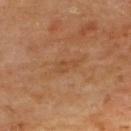follow-up: imaged on a skin check; not biopsied
illumination: cross-polarized illumination
automated metrics: an area of roughly 3.5 mm², an outline eccentricity of about 0.85 (0 = round, 1 = elongated), and two-axis asymmetry of about 0.35; an automated nevus-likeness rating near 0 out of 100 and lesion-presence confidence of about 100/100
image source: ~15 mm tile from a whole-body skin photo
site: the upper back
subject: male, roughly 65 years of age